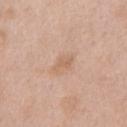Impression:
The lesion was photographed on a routine skin check and not biopsied; there is no pathology result.
Background:
A male subject, approximately 50 years of age. A 15 mm close-up tile from a total-body photography series done for melanoma screening. The lesion is located on the chest.